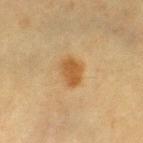The lesion was photographed on a routine skin check and not biopsied; there is no pathology result. A close-up tile cropped from a whole-body skin photograph, about 15 mm across. A female subject, roughly 55 years of age. The lesion is located on the left thigh. Longest diameter approximately 3 mm. Automated tile analysis of the lesion measured a lesion area of about 6.5 mm², a shape eccentricity near 0.65, and a shape-asymmetry score of about 0.2 (0 = symmetric). And it measured lesion-presence confidence of about 100/100.